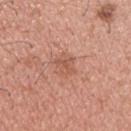Imaged during a routine full-body skin examination; the lesion was not biopsied and no histopathology is available.
On the upper back.
The recorded lesion diameter is about 3 mm.
The lesion-visualizer software estimated a border-irregularity index near 3.5/10, a color-variation rating of about 2.5/10, and radial color variation of about 1.
A 15 mm close-up tile from a total-body photography series done for melanoma screening.
The tile uses white-light illumination.
A male patient, roughly 35 years of age.